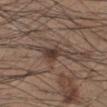Part of a total-body skin-imaging series; this lesion was reviewed on a skin check and was not flagged for biopsy.
The lesion is located on the left lower leg.
This image is a 15 mm lesion crop taken from a total-body photograph.
The tile uses white-light illumination.
Measured at roughly 4.5 mm in maximum diameter.
The patient is a male roughly 35 years of age.
Automated tile analysis of the lesion measured a border-irregularity rating of about 6/10, a color-variation rating of about 3/10, and peripheral color asymmetry of about 1. And it measured lesion-presence confidence of about 80/100.